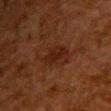Image and clinical context: A roughly 15 mm field-of-view crop from a total-body skin photograph. The tile uses cross-polarized illumination. The recorded lesion diameter is about 3.5 mm. On the chest. A female patient in their 50s. An algorithmic analysis of the crop reported a lesion–skin lightness drop of about 5 and a normalized lesion–skin contrast near 6.5. The analysis additionally found a border-irregularity index near 3.5/10, a within-lesion color-variation index near 2/10, and a peripheral color-asymmetry measure near 1. The analysis additionally found an automated nevus-likeness rating near 35 out of 100.